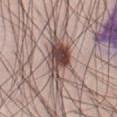Q: Is there a histopathology result?
A: no biopsy performed (imaged during a skin exam)
Q: What are the patient's age and sex?
A: male, about 35 years old
Q: Automated lesion metrics?
A: a footprint of about 13 mm², a shape eccentricity near 0.85, and a symmetry-axis asymmetry near 0.3; a color-variation rating of about 8/10 and radial color variation of about 2.5
Q: How was the tile lit?
A: white-light illumination
Q: What is the anatomic site?
A: the abdomen
Q: What kind of image is this?
A: ~15 mm crop, total-body skin-cancer survey